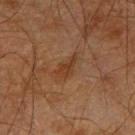Clinical impression: The lesion was photographed on a routine skin check and not biopsied; there is no pathology result. Acquisition and patient details: Cropped from a total-body skin-imaging series; the visible field is about 15 mm. Imaged with cross-polarized lighting. The patient is a male aged 63–67. From the right upper arm.